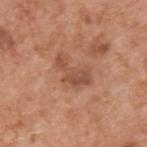Captured during whole-body skin photography for melanoma surveillance; the lesion was not biopsied.